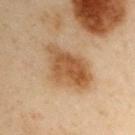This lesion was catalogued during total-body skin photography and was not selected for biopsy.